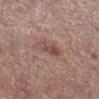Image and clinical context:
The lesion's longest dimension is about 3 mm. The patient is a male aged around 65. A 15 mm crop from a total-body photograph taken for skin-cancer surveillance. The tile uses white-light illumination. On the right lower leg.